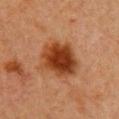Q: Was a biopsy performed?
A: catalogued during a skin exam; not biopsied
Q: What are the patient's age and sex?
A: female, about 40 years old
Q: What lighting was used for the tile?
A: cross-polarized illumination
Q: Lesion location?
A: the chest
Q: Lesion size?
A: ≈5 mm
Q: How was this image acquired?
A: ~15 mm tile from a whole-body skin photo